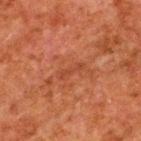| key | value |
|---|---|
| image-analysis metrics | a shape-asymmetry score of about 0.45 (0 = symmetric); a lesion–skin lightness drop of about 5 and a normalized border contrast of about 4.5; a border-irregularity index near 5.5/10, a color-variation rating of about 0/10, and radial color variation of about 0 |
| size | ≈3.5 mm |
| body site | the upper back |
| patient | male, aged approximately 80 |
| tile lighting | cross-polarized illumination |
| acquisition | ~15 mm crop, total-body skin-cancer survey |